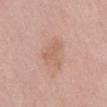Q: What is the imaging modality?
A: 15 mm crop, total-body photography
Q: What lighting was used for the tile?
A: white-light illumination
Q: Patient demographics?
A: male, approximately 60 years of age
Q: Automated lesion metrics?
A: a footprint of about 6 mm², an outline eccentricity of about 0.75 (0 = round, 1 = elongated), and two-axis asymmetry of about 0.6; a lesion color around L≈61 a*≈21 b*≈29 in CIELAB and a lesion–skin lightness drop of about 6; a detector confidence of about 100 out of 100 that the crop contains a lesion
Q: What is the anatomic site?
A: the front of the torso